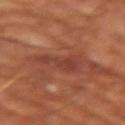Impression: The lesion was photographed on a routine skin check and not biopsied; there is no pathology result. Context: On the right upper arm. A lesion tile, about 15 mm wide, cut from a 3D total-body photograph. The subject is a male approximately 65 years of age.